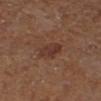Impression:
Part of a total-body skin-imaging series; this lesion was reviewed on a skin check and was not flagged for biopsy.
Clinical summary:
The patient is female. A lesion tile, about 15 mm wide, cut from a 3D total-body photograph. On the right lower leg.